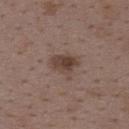Imaged during a routine full-body skin examination; the lesion was not biopsied and no histopathology is available. Approximately 4 mm at its widest. The lesion is located on the upper back. A 15 mm close-up tile from a total-body photography series done for melanoma screening. A female patient in their mid- to late 30s.